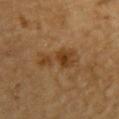Part of a total-body skin-imaging series; this lesion was reviewed on a skin check and was not flagged for biopsy.
A 15 mm close-up extracted from a 3D total-body photography capture.
An algorithmic analysis of the crop reported a footprint of about 10 mm², a shape eccentricity near 0.9, and two-axis asymmetry of about 0.4. And it measured a lesion color around L≈34 a*≈17 b*≈32 in CIELAB, roughly 7 lightness units darker than nearby skin, and a lesion-to-skin contrast of about 7 (normalized; higher = more distinct). It also reported a border-irregularity index near 5.5/10.
Located on the upper back.
A male subject approximately 85 years of age or older.